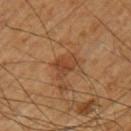The lesion was photographed on a routine skin check and not biopsied; there is no pathology result.
A male patient about 60 years old.
The lesion-visualizer software estimated a footprint of about 5.5 mm² and an eccentricity of roughly 0.65. The analysis additionally found about 7 CIELAB-L* units darker than the surrounding skin and a lesion-to-skin contrast of about 6.5 (normalized; higher = more distinct). And it measured an automated nevus-likeness rating near 5 out of 100 and lesion-presence confidence of about 100/100.
This image is a 15 mm lesion crop taken from a total-body photograph.
The lesion is located on the right upper arm.
This is a cross-polarized tile.